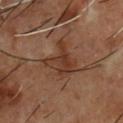Findings:
• follow-up — no biopsy performed (imaged during a skin exam)
• automated metrics — a lesion area of about 10 mm² and an outline eccentricity of about 0.55 (0 = round, 1 = elongated); a border-irregularity index near 6/10, a within-lesion color-variation index near 4/10, and peripheral color asymmetry of about 1.5; a nevus-likeness score of about 0/100 and a detector confidence of about 100 out of 100 that the crop contains a lesion
• acquisition — total-body-photography crop, ~15 mm field of view
• patient — male, approximately 55 years of age
• location — the front of the torso
• lesion diameter — about 4.5 mm
• tile lighting — cross-polarized